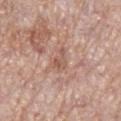The lesion was photographed on a routine skin check and not biopsied; there is no pathology result. A female subject aged 68 to 72. Automated image analysis of the tile measured an area of roughly 3.5 mm², a shape eccentricity near 0.75, and a shape-asymmetry score of about 0.5 (0 = symmetric). It also reported a classifier nevus-likeness of about 0/100 and lesion-presence confidence of about 80/100. This is a white-light tile. A region of skin cropped from a whole-body photographic capture, roughly 15 mm wide. On the leg.